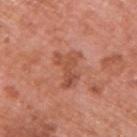Part of a total-body skin-imaging series; this lesion was reviewed on a skin check and was not flagged for biopsy. Longest diameter approximately 4.5 mm. A 15 mm close-up extracted from a 3D total-body photography capture. The lesion-visualizer software estimated an outline eccentricity of about 0.8 (0 = round, 1 = elongated) and a symmetry-axis asymmetry near 0.35. And it measured an average lesion color of about L≈52 a*≈27 b*≈33 (CIELAB) and about 8 CIELAB-L* units darker than the surrounding skin. A male subject, roughly 70 years of age. This is a white-light tile. From the right upper arm.